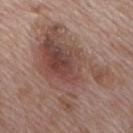Notes:
– workup — catalogued during a skin exam; not biopsied
– acquisition — ~15 mm tile from a whole-body skin photo
– size — about 10 mm
– patient — male, about 70 years old
– lighting — white-light illumination
– location — the mid back